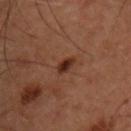This lesion was catalogued during total-body skin photography and was not selected for biopsy.
The subject is a male about 45 years old.
Measured at roughly 2.5 mm in maximum diameter.
This is a cross-polarized tile.
The lesion is on the chest.
An algorithmic analysis of the crop reported a footprint of about 3 mm², an eccentricity of roughly 0.75, and two-axis asymmetry of about 0.25. The software also gave a mean CIELAB color near L≈28 a*≈21 b*≈26 and a lesion–skin lightness drop of about 11. The analysis additionally found an automated nevus-likeness rating near 95 out of 100 and a detector confidence of about 100 out of 100 that the crop contains a lesion.
Cropped from a total-body skin-imaging series; the visible field is about 15 mm.